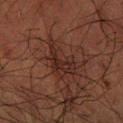Findings:
- follow-up · catalogued during a skin exam; not biopsied
- size · ≈5 mm
- site · the left forearm
- lighting · cross-polarized
- subject · male, approximately 60 years of age
- image · ~15 mm tile from a whole-body skin photo